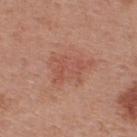Q: Is there a histopathology result?
A: imaged on a skin check; not biopsied
Q: How was the tile lit?
A: white-light illumination
Q: What are the patient's age and sex?
A: male, aged around 55
Q: What kind of image is this?
A: total-body-photography crop, ~15 mm field of view
Q: Lesion size?
A: about 5 mm
Q: Where on the body is the lesion?
A: the upper back